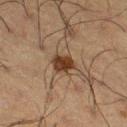<tbp_lesion>
  <biopsy_status>not biopsied; imaged during a skin examination</biopsy_status>
  <patient>
    <sex>male</sex>
    <age_approx>60</age_approx>
  </patient>
  <automated_metrics>
    <area_mm2_approx>5.5</area_mm2_approx>
    <eccentricity>0.6</eccentricity>
    <shape_asymmetry>0.25</shape_asymmetry>
    <cielab_L>29</cielab_L>
    <cielab_a>15</cielab_a>
    <cielab_b>25</cielab_b>
    <vs_skin_darker_L>11.0</vs_skin_darker_L>
    <vs_skin_contrast_norm>11.0</vs_skin_contrast_norm>
    <border_irregularity_0_10>2.0</border_irregularity_0_10>
    <color_variation_0_10>3.0</color_variation_0_10>
    <peripheral_color_asymmetry>1.0</peripheral_color_asymmetry>
    <nevus_likeness_0_100>95</nevus_likeness_0_100>
    <lesion_detection_confidence_0_100>100</lesion_detection_confidence_0_100>
  </automated_metrics>
  <site>right thigh</site>
  <lighting>cross-polarized</lighting>
  <image>
    <source>total-body photography crop</source>
    <field_of_view_mm>15</field_of_view_mm>
  </image>
  <lesion_size>
    <long_diameter_mm_approx>3.0</long_diameter_mm_approx>
  </lesion_size>
</tbp_lesion>